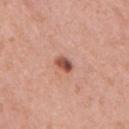Case summary:
* workup: total-body-photography surveillance lesion; no biopsy
* subject: female, approximately 40 years of age
* size: ≈2.5 mm
* location: the right upper arm
* lighting: white-light
* acquisition: 15 mm crop, total-body photography